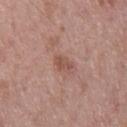Q: Was a biopsy performed?
A: imaged on a skin check; not biopsied
Q: How was the tile lit?
A: white-light illumination
Q: What did automated image analysis measure?
A: an area of roughly 3.5 mm², an outline eccentricity of about 0.8 (0 = round, 1 = elongated), and a shape-asymmetry score of about 0.15 (0 = symmetric)
Q: What is the imaging modality?
A: ~15 mm crop, total-body skin-cancer survey
Q: Who is the patient?
A: male, roughly 50 years of age
Q: What is the anatomic site?
A: the right thigh
Q: Lesion size?
A: about 2.5 mm